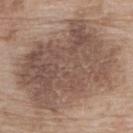Imaged during a routine full-body skin examination; the lesion was not biopsied and no histopathology is available.
A female patient aged 73 to 77.
Located on the upper back.
This is a white-light tile.
A 15 mm close-up tile from a total-body photography series done for melanoma screening.
About 12 mm across.